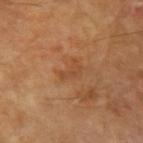This lesion was catalogued during total-body skin photography and was not selected for biopsy. Automated tile analysis of the lesion measured a shape eccentricity near 0.85 and a symmetry-axis asymmetry near 0.5. The analysis additionally found a mean CIELAB color near L≈45 a*≈22 b*≈35, a lesion–skin lightness drop of about 6, and a normalized border contrast of about 5. It also reported a nevus-likeness score of about 0/100. Located on the arm. A 15 mm crop from a total-body photograph taken for skin-cancer surveillance. The tile uses cross-polarized illumination. The recorded lesion diameter is about 3 mm. A male subject, roughly 70 years of age.